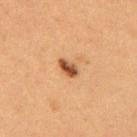Recorded during total-body skin imaging; not selected for excision or biopsy.
The lesion-visualizer software estimated a footprint of about 3.5 mm², an outline eccentricity of about 0.85 (0 = round, 1 = elongated), and a shape-asymmetry score of about 0.2 (0 = symmetric). It also reported a mean CIELAB color near L≈43 a*≈21 b*≈33 and roughly 14 lightness units darker than nearby skin. The software also gave a border-irregularity rating of about 2/10, internal color variation of about 3.5 on a 0–10 scale, and radial color variation of about 1.5. And it measured a nevus-likeness score of about 95/100 and a detector confidence of about 100 out of 100 that the crop contains a lesion.
Cropped from a whole-body photographic skin survey; the tile spans about 15 mm.
On the upper back.
A female patient aged 38–42.
Measured at roughly 2.5 mm in maximum diameter.